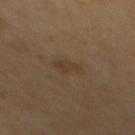The lesion was tiled from a total-body skin photograph and was not biopsied. The lesion is on the upper back. Approximately 2.5 mm at its widest. A 15 mm close-up tile from a total-body photography series done for melanoma screening. Imaged with cross-polarized lighting. A female patient, aged approximately 60.